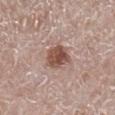A close-up tile cropped from a whole-body skin photograph, about 15 mm across.
A female subject, aged 48 to 52.
The lesion-visualizer software estimated a border-irregularity index near 2/10, a color-variation rating of about 4.5/10, and peripheral color asymmetry of about 1.5. The software also gave a nevus-likeness score of about 95/100 and lesion-presence confidence of about 100/100.
This is a white-light tile.
The lesion is located on the right lower leg.